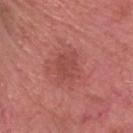Assessment:
Captured during whole-body skin photography for melanoma surveillance; the lesion was not biopsied.
Background:
The tile uses white-light illumination. A roughly 15 mm field-of-view crop from a total-body skin photograph. An algorithmic analysis of the crop reported a lesion color around L≈48 a*≈30 b*≈26 in CIELAB and a lesion–skin lightness drop of about 7. The lesion is on the head or neck. A male patient aged 63–67.